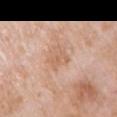A female subject, about 70 years old.
Cropped from a whole-body photographic skin survey; the tile spans about 15 mm.
From the right upper arm.